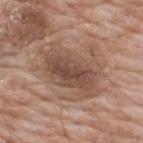Findings:
• biopsy status: catalogued during a skin exam; not biopsied
• illumination: white-light illumination
• automated metrics: an average lesion color of about L≈50 a*≈18 b*≈26 (CIELAB), roughly 10 lightness units darker than nearby skin, and a normalized lesion–skin contrast near 7.5; a border-irregularity rating of about 3.5/10 and a within-lesion color-variation index near 5/10
• site: the upper back
• subject: male, in their 60s
• lesion diameter: ~7.5 mm (longest diameter)
• image: total-body-photography crop, ~15 mm field of view